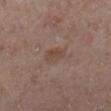Part of a total-body skin-imaging series; this lesion was reviewed on a skin check and was not flagged for biopsy. The lesion is on the right lower leg. Automated tile analysis of the lesion measured a lesion color around L≈45 a*≈17 b*≈25 in CIELAB, a lesion–skin lightness drop of about 6, and a normalized lesion–skin contrast near 6. It also reported a border-irregularity index near 2/10 and a within-lesion color-variation index near 2/10. The tile uses white-light illumination. The patient is a female approximately 40 years of age. A lesion tile, about 15 mm wide, cut from a 3D total-body photograph. Approximately 3 mm at its widest.